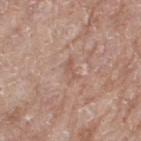workup — no biopsy performed (imaged during a skin exam)
imaging modality — ~15 mm tile from a whole-body skin photo
site — the right thigh
diameter — ≈2.5 mm
subject — female, in their mid- to late 70s
illumination — white-light